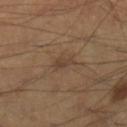Context:
This is a cross-polarized tile. The recorded lesion diameter is about 2.5 mm. On the right lower leg. The total-body-photography lesion software estimated a lesion color around L≈40 a*≈16 b*≈26 in CIELAB and a lesion-to-skin contrast of about 6 (normalized; higher = more distinct). A male patient about 40 years old. A 15 mm crop from a total-body photograph taken for skin-cancer surveillance.